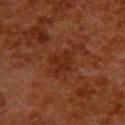Findings:
• notes · no biopsy performed (imaged during a skin exam)
• lighting · cross-polarized illumination
• image · 15 mm crop, total-body photography
• patient · female, aged around 50
• anatomic site · the upper back
• size · ~3 mm (longest diameter)